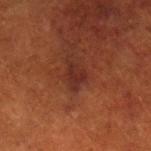Captured during whole-body skin photography for melanoma surveillance; the lesion was not biopsied. Automated image analysis of the tile measured a lesion area of about 4.5 mm², an eccentricity of roughly 0.75, and a symmetry-axis asymmetry near 0.4. It also reported a nevus-likeness score of about 5/100 and a detector confidence of about 90 out of 100 that the crop contains a lesion. The subject is a female aged 78–82. The lesion is located on the left lower leg. The tile uses cross-polarized illumination. Cropped from a total-body skin-imaging series; the visible field is about 15 mm.